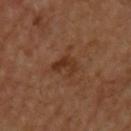notes: imaged on a skin check; not biopsied | lesion diameter: about 3 mm | imaging modality: ~15 mm tile from a whole-body skin photo | body site: the upper back.